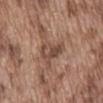Recorded during total-body skin imaging; not selected for excision or biopsy. Imaged with white-light lighting. The subject is a male aged 73 to 77. The lesion is on the abdomen. A roughly 15 mm field-of-view crop from a total-body skin photograph.